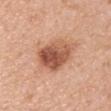Assessment:
Captured during whole-body skin photography for melanoma surveillance; the lesion was not biopsied.
Background:
Approximately 5.5 mm at its widest. A female subject aged around 50. The lesion is located on the right upper arm. Cropped from a whole-body photographic skin survey; the tile spans about 15 mm. The tile uses white-light illumination. The lesion-visualizer software estimated a shape eccentricity near 0.7 and a symmetry-axis asymmetry near 0.2.